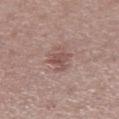Impression:
Recorded during total-body skin imaging; not selected for excision or biopsy.
Context:
The lesion is on the right lower leg. The tile uses white-light illumination. Measured at roughly 3 mm in maximum diameter. Automated image analysis of the tile measured a footprint of about 6.5 mm² and two-axis asymmetry of about 0.4. The analysis additionally found a border-irregularity rating of about 5.5/10, a color-variation rating of about 2.5/10, and radial color variation of about 1. And it measured an automated nevus-likeness rating near 10 out of 100. A female patient aged 48–52. A 15 mm close-up extracted from a 3D total-body photography capture.